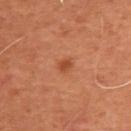Q: Was a biopsy performed?
A: total-body-photography surveillance lesion; no biopsy
Q: Lesion size?
A: about 1.5 mm
Q: Lesion location?
A: the back
Q: What is the imaging modality?
A: 15 mm crop, total-body photography
Q: Automated lesion metrics?
A: a border-irregularity rating of about 2/10, a color-variation rating of about 1/10, and radial color variation of about 0.5
Q: Patient demographics?
A: male, approximately 50 years of age